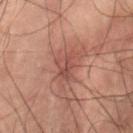Q: Was a biopsy performed?
A: no biopsy performed (imaged during a skin exam)
Q: What is the imaging modality?
A: ~15 mm crop, total-body skin-cancer survey
Q: Where on the body is the lesion?
A: the abdomen
Q: How was the tile lit?
A: cross-polarized illumination
Q: Lesion size?
A: ≈3.5 mm
Q: What are the patient's age and sex?
A: male, aged approximately 60